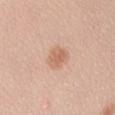• notes · total-body-photography surveillance lesion; no biopsy
• illumination · white-light
• site · the left forearm
• lesion diameter · about 2.5 mm
• subject · female, aged 23–27
• image · ~15 mm crop, total-body skin-cancer survey